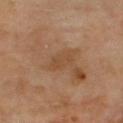This lesion was catalogued during total-body skin photography and was not selected for biopsy. Approximately 3 mm at its widest. A close-up tile cropped from a whole-body skin photograph, about 15 mm across. From the chest. A male subject, roughly 70 years of age.